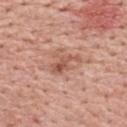Case summary:
* follow-up: catalogued during a skin exam; not biopsied
* body site: the upper back
* patient: male, about 60 years old
* imaging modality: ~15 mm tile from a whole-body skin photo